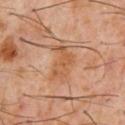Notes:
* follow-up: no biopsy performed (imaged during a skin exam)
* acquisition: ~15 mm tile from a whole-body skin photo
* diameter: ≈5 mm
* patient: male, about 60 years old
* site: the chest